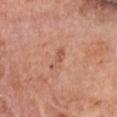Captured during whole-body skin photography for melanoma surveillance; the lesion was not biopsied. A close-up tile cropped from a whole-body skin photograph, about 15 mm across. The subject is a female roughly 60 years of age. The lesion is on the chest.